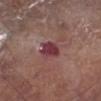follow-up = total-body-photography surveillance lesion; no biopsy
patient = male, in their 80s
site = the leg
image = 15 mm crop, total-body photography